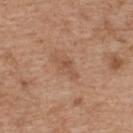Clinical impression: Recorded during total-body skin imaging; not selected for excision or biopsy. Context: A 15 mm crop from a total-body photograph taken for skin-cancer surveillance. A female subject, roughly 40 years of age. The tile uses white-light illumination. Located on the upper back. About 3.5 mm across.